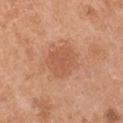This lesion was catalogued during total-body skin photography and was not selected for biopsy.
The lesion-visualizer software estimated border irregularity of about 2 on a 0–10 scale, a color-variation rating of about 2/10, and peripheral color asymmetry of about 0.5. The software also gave an automated nevus-likeness rating near 45 out of 100.
This is a white-light tile.
Approximately 4 mm at its widest.
Located on the arm.
A male patient, aged 53–57.
A lesion tile, about 15 mm wide, cut from a 3D total-body photograph.